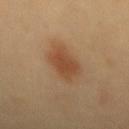Notes:
- biopsy status · no biopsy performed (imaged during a skin exam)
- subject · female, aged 43–47
- imaging modality · total-body-photography crop, ~15 mm field of view
- anatomic site · the mid back